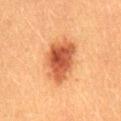The lesion was photographed on a routine skin check and not biopsied; there is no pathology result.
Automated tile analysis of the lesion measured a lesion area of about 16 mm², a shape eccentricity near 0.75, and two-axis asymmetry of about 0.2. The software also gave peripheral color asymmetry of about 1.5. It also reported a lesion-detection confidence of about 100/100.
Approximately 6 mm at its widest.
This is a cross-polarized tile.
A close-up tile cropped from a whole-body skin photograph, about 15 mm across.
On the lower back.
A male subject approximately 50 years of age.